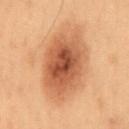Recorded during total-body skin imaging; not selected for excision or biopsy. The lesion is on the mid back. Imaged with cross-polarized lighting. A 15 mm close-up extracted from a 3D total-body photography capture. A male patient, about 55 years old. Automated tile analysis of the lesion measured an outline eccentricity of about 0.85 (0 = round, 1 = elongated) and a symmetry-axis asymmetry near 0.15. The software also gave a border-irregularity index near 2.5/10, internal color variation of about 6.5 on a 0–10 scale, and radial color variation of about 2.